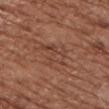Clinical impression: Imaged during a routine full-body skin examination; the lesion was not biopsied and no histopathology is available. Image and clinical context: A female patient, in their mid- to late 70s. The tile uses white-light illumination. The recorded lesion diameter is about 4.5 mm. A 15 mm close-up tile from a total-body photography series done for melanoma screening. On the left upper arm. An algorithmic analysis of the crop reported a nevus-likeness score of about 0/100 and a detector confidence of about 70 out of 100 that the crop contains a lesion.